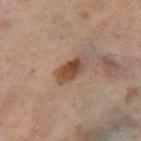Automated image analysis of the tile measured an average lesion color of about L≈48 a*≈20 b*≈30 (CIELAB) and a lesion–skin lightness drop of about 12. The analysis additionally found an automated nevus-likeness rating near 90 out of 100 and lesion-presence confidence of about 100/100.
The patient is a female in their mid-50s.
Approximately 4 mm at its widest.
A close-up tile cropped from a whole-body skin photograph, about 15 mm across.
The lesion is on the right thigh.
This is a cross-polarized tile.